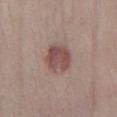notes: imaged on a skin check; not biopsied
image-analysis metrics: border irregularity of about 2 on a 0–10 scale and a within-lesion color-variation index near 3.5/10
subject: female, aged 38 to 42
image source: total-body-photography crop, ~15 mm field of view
tile lighting: white-light illumination
anatomic site: the leg